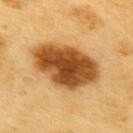No biopsy was performed on this lesion — it was imaged during a full skin examination and was not determined to be concerning.
The subject is a male in their 60s.
Captured under cross-polarized illumination.
Measured at roughly 8 mm in maximum diameter.
A roughly 15 mm field-of-view crop from a total-body skin photograph.
The lesion is on the upper back.